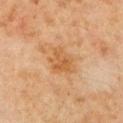Clinical impression: Part of a total-body skin-imaging series; this lesion was reviewed on a skin check and was not flagged for biopsy. Background: The total-body-photography lesion software estimated a footprint of about 7.5 mm² and an eccentricity of roughly 0.6. The analysis additionally found an average lesion color of about L≈54 a*≈21 b*≈40 (CIELAB), about 7 CIELAB-L* units darker than the surrounding skin, and a normalized border contrast of about 6. The software also gave a border-irregularity rating of about 3.5/10, a within-lesion color-variation index near 3/10, and peripheral color asymmetry of about 1. And it measured a nevus-likeness score of about 10/100 and a detector confidence of about 100 out of 100 that the crop contains a lesion. A region of skin cropped from a whole-body photographic capture, roughly 15 mm wide. A male subject, aged 58–62. The lesion's longest dimension is about 3.5 mm. The lesion is located on the left upper arm.